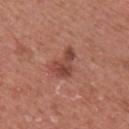A male subject in their mid-40s. The lesion is on the left upper arm. A roughly 15 mm field-of-view crop from a total-body skin photograph.